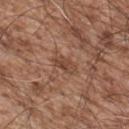The lesion's longest dimension is about 4 mm.
Automated tile analysis of the lesion measured a footprint of about 5.5 mm², an outline eccentricity of about 0.9 (0 = round, 1 = elongated), and a shape-asymmetry score of about 0.3 (0 = symmetric). And it measured a border-irregularity rating of about 3.5/10, internal color variation of about 3 on a 0–10 scale, and a peripheral color-asymmetry measure near 1. It also reported lesion-presence confidence of about 95/100.
A roughly 15 mm field-of-view crop from a total-body skin photograph.
A male patient, roughly 55 years of age.
This is a white-light tile.
On the back.